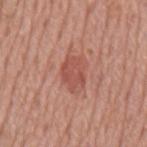Clinical impression: Recorded during total-body skin imaging; not selected for excision or biopsy. Background: A region of skin cropped from a whole-body photographic capture, roughly 15 mm wide. The patient is a male aged approximately 75. Located on the mid back.